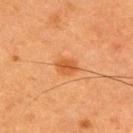The lesion was tiled from a total-body skin photograph and was not biopsied.
Cropped from a total-body skin-imaging series; the visible field is about 15 mm.
Located on the upper back.
A male subject aged approximately 50.